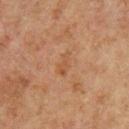Impression: Captured during whole-body skin photography for melanoma surveillance; the lesion was not biopsied. Acquisition and patient details: A roughly 15 mm field-of-view crop from a total-body skin photograph. Imaged with cross-polarized lighting. An algorithmic analysis of the crop reported a classifier nevus-likeness of about 0/100 and a detector confidence of about 100 out of 100 that the crop contains a lesion. The lesion is located on the front of the torso. Measured at roughly 3 mm in maximum diameter. A male patient aged around 60.